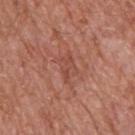The lesion was photographed on a routine skin check and not biopsied; there is no pathology result.
A 15 mm close-up extracted from a 3D total-body photography capture.
Located on the upper back.
The subject is a male aged 63–67.
Captured under white-light illumination.